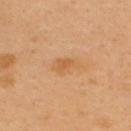No biopsy was performed on this lesion — it was imaged during a full skin examination and was not determined to be concerning.
On the upper back.
Approximately 3.5 mm at its widest.
Captured under cross-polarized illumination.
A 15 mm crop from a total-body photograph taken for skin-cancer surveillance.
The subject is a male aged 38–42.
The lesion-visualizer software estimated a footprint of about 5 mm² and an outline eccentricity of about 0.85 (0 = round, 1 = elongated). It also reported a lesion color around L≈60 a*≈22 b*≈41 in CIELAB, about 7 CIELAB-L* units darker than the surrounding skin, and a normalized border contrast of about 5.5.